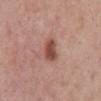Background: The lesion-visualizer software estimated an area of roughly 6 mm² and a symmetry-axis asymmetry near 0.2. The analysis additionally found roughly 13 lightness units darker than nearby skin and a normalized border contrast of about 9. And it measured a border-irregularity rating of about 2/10 and a peripheral color-asymmetry measure near 1.5. And it measured an automated nevus-likeness rating near 85 out of 100 and a lesion-detection confidence of about 100/100. On the mid back. A close-up tile cropped from a whole-body skin photograph, about 15 mm across. Measured at roughly 3.5 mm in maximum diameter. The patient is a male about 55 years old.